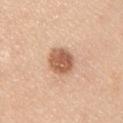Impression:
Captured during whole-body skin photography for melanoma surveillance; the lesion was not biopsied.
Background:
A male patient roughly 35 years of age. Approximately 4 mm at its widest. The lesion-visualizer software estimated a lesion area of about 8.5 mm² and a shape-asymmetry score of about 0.2 (0 = symmetric). It also reported an automated nevus-likeness rating near 75 out of 100 and a lesion-detection confidence of about 100/100. This image is a 15 mm lesion crop taken from a total-body photograph. On the left upper arm. Imaged with white-light lighting.